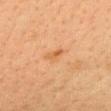The lesion was photographed on a routine skin check and not biopsied; there is no pathology result. A close-up tile cropped from a whole-body skin photograph, about 15 mm across. This is a cross-polarized tile. The subject is a female aged 38 to 42. On the head or neck. The total-body-photography lesion software estimated a footprint of about 3.5 mm², an outline eccentricity of about 0.8 (0 = round, 1 = elongated), and a shape-asymmetry score of about 0.4 (0 = symmetric). The software also gave a lesion-to-skin contrast of about 5 (normalized; higher = more distinct). And it measured a border-irregularity index near 3.5/10, internal color variation of about 3 on a 0–10 scale, and peripheral color asymmetry of about 1. The software also gave a nevus-likeness score of about 0/100 and lesion-presence confidence of about 100/100. Approximately 3 mm at its widest.